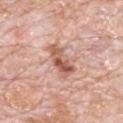Imaged during a routine full-body skin examination; the lesion was not biopsied and no histopathology is available.
Longest diameter approximately 4.5 mm.
A 15 mm close-up extracted from a 3D total-body photography capture.
Located on the mid back.
This is a white-light tile.
A male patient, aged approximately 80.